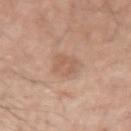Case summary:
* biopsy status: no biopsy performed (imaged during a skin exam)
* patient: male, aged 53 to 57
* image: ~15 mm tile from a whole-body skin photo
* location: the left upper arm